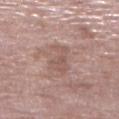Q: Was this lesion biopsied?
A: imaged on a skin check; not biopsied
Q: How was the tile lit?
A: white-light illumination
Q: Who is the patient?
A: female, in their 70s
Q: Lesion location?
A: the right lower leg
Q: How large is the lesion?
A: ≈4.5 mm
Q: How was this image acquired?
A: ~15 mm tile from a whole-body skin photo
Q: Automated lesion metrics?
A: a footprint of about 7 mm², a shape eccentricity near 0.9, and a symmetry-axis asymmetry near 0.4; an automated nevus-likeness rating near 0 out of 100